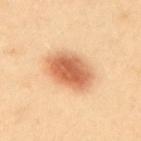| field | value |
|---|---|
| biopsy status | imaged on a skin check; not biopsied |
| subject | female, aged 38–42 |
| automated metrics | a normalized lesion–skin contrast near 9.5; a color-variation rating of about 6/10 and peripheral color asymmetry of about 1.5; a classifier nevus-likeness of about 100/100 and a detector confidence of about 100 out of 100 that the crop contains a lesion |
| location | the upper back |
| tile lighting | cross-polarized illumination |
| size | ≈5.5 mm |
| image source | total-body-photography crop, ~15 mm field of view |